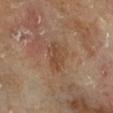Acquisition and patient details: Cropped from a whole-body photographic skin survey; the tile spans about 15 mm. About 3.5 mm across. The subject is a male aged 63–67. The lesion-visualizer software estimated a lesion color around L≈46 a*≈19 b*≈30 in CIELAB and a normalized lesion–skin contrast near 5.5. And it measured a border-irregularity rating of about 2.5/10 and peripheral color asymmetry of about 1. Imaged with cross-polarized lighting. From the right lower leg.